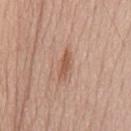Clinical impression: The lesion was tiled from a total-body skin photograph and was not biopsied. Background: The subject is a male aged approximately 55. This is a white-light tile. A 15 mm close-up extracted from a 3D total-body photography capture. Automated image analysis of the tile measured a lesion area of about 3.5 mm². The analysis additionally found a lesion color around L≈55 a*≈22 b*≈30 in CIELAB, roughly 10 lightness units darker than nearby skin, and a normalized lesion–skin contrast near 7.5. It also reported an automated nevus-likeness rating near 55 out of 100 and a detector confidence of about 100 out of 100 that the crop contains a lesion. About 3.5 mm across.